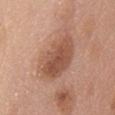{"site": "front of the torso", "image": {"source": "total-body photography crop", "field_of_view_mm": 15}, "patient": {"sex": "female", "age_approx": 60}}